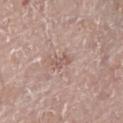Recorded during total-body skin imaging; not selected for excision or biopsy. Cropped from a total-body skin-imaging series; the visible field is about 15 mm. The lesion-visualizer software estimated a lesion area of about 3 mm² and a symmetry-axis asymmetry near 0.25. The analysis additionally found an average lesion color of about L≈57 a*≈18 b*≈23 (CIELAB) and a normalized lesion–skin contrast near 5.5. And it measured lesion-presence confidence of about 100/100. A male patient in their 70s. Imaged with white-light lighting. On the right leg.